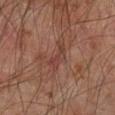The lesion was photographed on a routine skin check and not biopsied; there is no pathology result. Located on the left forearm. A 15 mm close-up extracted from a 3D total-body photography capture. Captured under cross-polarized illumination. A male subject aged approximately 45. The lesion's longest dimension is about 3.5 mm.